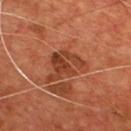Case summary:
– follow-up · imaged on a skin check; not biopsied
– subject · male, aged around 55
– site · the chest
– image-analysis metrics · a lesion area of about 12 mm², an eccentricity of roughly 0.7, and two-axis asymmetry of about 0.45; border irregularity of about 6.5 on a 0–10 scale, a within-lesion color-variation index near 4.5/10, and a peripheral color-asymmetry measure near 1.5; a nevus-likeness score of about 0/100 and lesion-presence confidence of about 100/100
– lesion size · ~5.5 mm (longest diameter)
– image source · ~15 mm crop, total-body skin-cancer survey
– lighting · cross-polarized